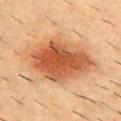workup = catalogued during a skin exam; not biopsied | subject = male, aged 53 to 57 | imaging modality = ~15 mm tile from a whole-body skin photo | anatomic site = the upper back.